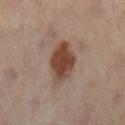{"biopsy_status": "not biopsied; imaged during a skin examination", "site": "left leg", "automated_metrics": {"eccentricity": 0.7, "shape_asymmetry": 0.2, "nevus_likeness_0_100": 95}, "lesion_size": {"long_diameter_mm_approx": 5.0}, "patient": {"sex": "female", "age_approx": 60}, "lighting": "cross-polarized", "image": {"source": "total-body photography crop", "field_of_view_mm": 15}}